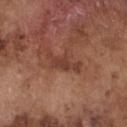A 15 mm close-up tile from a total-body photography series done for melanoma screening.
The lesion is on the chest.
Captured under white-light illumination.
Measured at roughly 4.5 mm in maximum diameter.
A male patient aged 73–77.